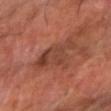The lesion was tiled from a total-body skin photograph and was not biopsied. This is a cross-polarized tile. Approximately 4.5 mm at its widest. An algorithmic analysis of the crop reported a mean CIELAB color near L≈41 a*≈24 b*≈29 and roughly 7 lightness units darker than nearby skin. And it measured border irregularity of about 6.5 on a 0–10 scale, internal color variation of about 4 on a 0–10 scale, and a peripheral color-asymmetry measure near 1. And it measured a classifier nevus-likeness of about 0/100. The lesion is located on the right forearm. Cropped from a total-body skin-imaging series; the visible field is about 15 mm. The subject is aged 63–67.